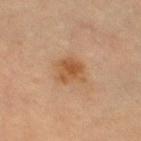{
  "biopsy_status": "not biopsied; imaged during a skin examination",
  "patient": {
    "sex": "female",
    "age_approx": 70
  },
  "automated_metrics": {
    "eccentricity": 0.4,
    "shape_asymmetry": 0.2,
    "border_irregularity_0_10": 2.5,
    "color_variation_0_10": 3.5,
    "peripheral_color_asymmetry": 1.0
  },
  "lesion_size": {
    "long_diameter_mm_approx": 3.0
  },
  "image": {
    "source": "total-body photography crop",
    "field_of_view_mm": 15
  },
  "site": "left thigh",
  "lighting": "cross-polarized"
}